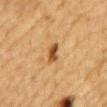Assessment:
No biopsy was performed on this lesion — it was imaged during a full skin examination and was not determined to be concerning.
Image and clinical context:
The recorded lesion diameter is about 2.5 mm. Automated tile analysis of the lesion measured an automated nevus-likeness rating near 80 out of 100 and a lesion-detection confidence of about 100/100. A 15 mm close-up tile from a total-body photography series done for melanoma screening. Captured under cross-polarized illumination. From the back. The patient is a male aged approximately 85 or older.